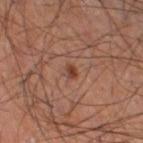biopsy status = catalogued during a skin exam; not biopsied
image source = total-body-photography crop, ~15 mm field of view
location = the right thigh
subject = male, aged 63–67